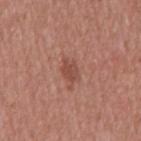Clinical impression: No biopsy was performed on this lesion — it was imaged during a full skin examination and was not determined to be concerning. Context: Cropped from a total-body skin-imaging series; the visible field is about 15 mm. The lesion is on the chest. The subject is a male aged around 45. The tile uses white-light illumination. The recorded lesion diameter is about 3 mm.